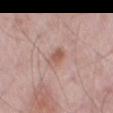Assessment: The lesion was photographed on a routine skin check and not biopsied; there is no pathology result. Context: The subject is a male aged approximately 55. A 15 mm crop from a total-body photograph taken for skin-cancer surveillance. The lesion is located on the left thigh.